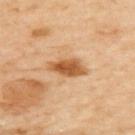image:
  source: total-body photography crop
  field_of_view_mm: 15
site: upper back
lesion_size:
  long_diameter_mm_approx: 4.5
patient:
  sex: female
  age_approx: 60